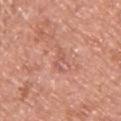Captured during whole-body skin photography for melanoma surveillance; the lesion was not biopsied. From the chest. About 3 mm across. Captured under white-light illumination. A male patient, aged approximately 55. An algorithmic analysis of the crop reported a footprint of about 3.5 mm², an eccentricity of roughly 0.85, and two-axis asymmetry of about 0.55. The software also gave a classifier nevus-likeness of about 0/100 and lesion-presence confidence of about 90/100. Cropped from a whole-body photographic skin survey; the tile spans about 15 mm.